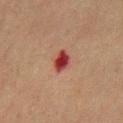follow-up — catalogued during a skin exam; not biopsied | lesion size — about 3 mm | image — ~15 mm tile from a whole-body skin photo | location — the chest | lighting — cross-polarized | patient — male, approximately 65 years of age.